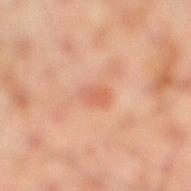  biopsy_status: not biopsied; imaged during a skin examination
  patient:
    sex: male
    age_approx: 45
  automated_metrics:
    border_irregularity_0_10: 3.0
    color_variation_0_10: 3.0
    peripheral_color_asymmetry: 1.0
    nevus_likeness_0_100: 0
    lesion_detection_confidence_0_100: 100
  image:
    source: total-body photography crop
    field_of_view_mm: 15
  lighting: cross-polarized
  lesion_size:
    long_diameter_mm_approx: 3.0
  site: right lower leg